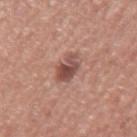Part of a total-body skin-imaging series; this lesion was reviewed on a skin check and was not flagged for biopsy. From the mid back. A 15 mm close-up tile from a total-body photography series done for melanoma screening. A male patient in their mid- to late 70s.